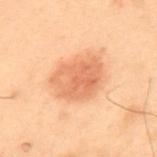Q: Was a biopsy performed?
A: no biopsy performed (imaged during a skin exam)
Q: Patient demographics?
A: male, aged around 55
Q: Where on the body is the lesion?
A: the mid back
Q: Lesion size?
A: ~5.5 mm (longest diameter)
Q: What kind of image is this?
A: ~15 mm crop, total-body skin-cancer survey
Q: Automated lesion metrics?
A: a lesion area of about 18 mm² and two-axis asymmetry of about 0.2